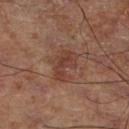Clinical impression:
Imaged during a routine full-body skin examination; the lesion was not biopsied and no histopathology is available.
Clinical summary:
Automated tile analysis of the lesion measured a footprint of about 7 mm², an outline eccentricity of about 0.85 (0 = round, 1 = elongated), and a symmetry-axis asymmetry near 0.4. It also reported about 8 CIELAB-L* units darker than the surrounding skin. And it measured border irregularity of about 4.5 on a 0–10 scale, a color-variation rating of about 2.5/10, and radial color variation of about 0.5. The analysis additionally found an automated nevus-likeness rating near 25 out of 100. Captured under cross-polarized illumination. About 4 mm across. Located on the right thigh. A 15 mm close-up tile from a total-body photography series done for melanoma screening.